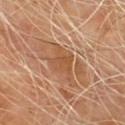Impression:
The lesion was tiled from a total-body skin photograph and was not biopsied.
Image and clinical context:
A close-up tile cropped from a whole-body skin photograph, about 15 mm across. The lesion is located on the chest. Imaged with cross-polarized lighting. Longest diameter approximately 4 mm. The lesion-visualizer software estimated a footprint of about 7.5 mm², an eccentricity of roughly 0.75, and a symmetry-axis asymmetry near 0.4. It also reported a lesion color around L≈51 a*≈22 b*≈36 in CIELAB, about 8 CIELAB-L* units darker than the surrounding skin, and a normalized border contrast of about 6.5. A male subject, aged around 70.